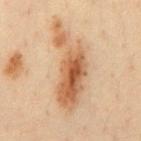Captured during whole-body skin photography for melanoma surveillance; the lesion was not biopsied. The patient is a male aged approximately 35. A close-up tile cropped from a whole-body skin photograph, about 15 mm across. On the front of the torso.